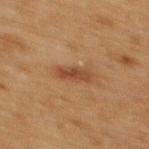– biopsy status — catalogued during a skin exam; not biopsied
– tile lighting — cross-polarized illumination
– subject — male, approximately 50 years of age
– lesion diameter — ~3.5 mm (longest diameter)
– site — the mid back
– imaging modality — total-body-photography crop, ~15 mm field of view